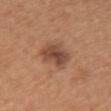{
  "biopsy_status": "not biopsied; imaged during a skin examination",
  "site": "chest",
  "image": {
    "source": "total-body photography crop",
    "field_of_view_mm": 15
  },
  "patient": {
    "sex": "female",
    "age_approx": 55
  },
  "automated_metrics": {
    "cielab_L": 47,
    "cielab_a": 21,
    "cielab_b": 30,
    "vs_skin_darker_L": 11.0
  }
}